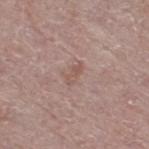{
  "automated_metrics": {
    "border_irregularity_0_10": 3.5,
    "color_variation_0_10": 0.0,
    "lesion_detection_confidence_0_100": 100
  },
  "lesion_size": {
    "long_diameter_mm_approx": 2.5
  },
  "patient": {
    "sex": "female",
    "age_approx": 65
  },
  "lighting": "white-light",
  "site": "leg",
  "image": {
    "source": "total-body photography crop",
    "field_of_view_mm": 15
  }
}